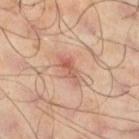<tbp_lesion>
<biopsy_status>not biopsied; imaged during a skin examination</biopsy_status>
<patient>
  <sex>male</sex>
  <age_approx>65</age_approx>
</patient>
<image>
  <source>total-body photography crop</source>
  <field_of_view_mm>15</field_of_view_mm>
</image>
<lesion_size>
  <long_diameter_mm_approx>3.5</long_diameter_mm_approx>
</lesion_size>
<site>left lower leg</site>
</tbp_lesion>